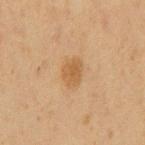Q: Is there a histopathology result?
A: no biopsy performed (imaged during a skin exam)
Q: What are the patient's age and sex?
A: male, aged 58 to 62
Q: How large is the lesion?
A: ≈3 mm
Q: What is the anatomic site?
A: the back
Q: What kind of image is this?
A: total-body-photography crop, ~15 mm field of view
Q: What did automated image analysis measure?
A: a footprint of about 5.5 mm², a shape eccentricity near 0.7, and two-axis asymmetry of about 0.2; a mean CIELAB color near L≈47 a*≈16 b*≈34, a lesion–skin lightness drop of about 7, and a normalized border contrast of about 6.5; a nevus-likeness score of about 55/100 and lesion-presence confidence of about 100/100